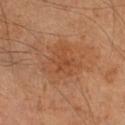workup — catalogued during a skin exam; not biopsied | acquisition — ~15 mm tile from a whole-body skin photo | illumination — cross-polarized | subject — male, aged 63 to 67 | automated metrics — a footprint of about 13 mm², a shape eccentricity near 0.4, and a shape-asymmetry score of about 0.35 (0 = symmetric); a lesion color around L≈47 a*≈23 b*≈34 in CIELAB and a normalized lesion–skin contrast near 5; border irregularity of about 4 on a 0–10 scale, a color-variation rating of about 3/10, and radial color variation of about 1; a classifier nevus-likeness of about 5/100 and lesion-presence confidence of about 100/100 | site — the right lower leg.